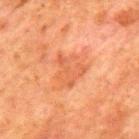Q: Was a biopsy performed?
A: total-body-photography surveillance lesion; no biopsy
Q: Patient demographics?
A: male, aged approximately 80
Q: How was the tile lit?
A: cross-polarized
Q: What kind of image is this?
A: 15 mm crop, total-body photography
Q: What is the anatomic site?
A: the mid back